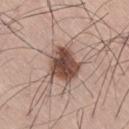  biopsy_status: not biopsied; imaged during a skin examination
  lesion_size:
    long_diameter_mm_approx: 4.0
  lighting: white-light
  patient:
    sex: male
    age_approx: 60
  site: leg
  image:
    source: total-body photography crop
    field_of_view_mm: 15
  automated_metrics:
    area_mm2_approx: 13.0
    shape_asymmetry: 0.25
    peripheral_color_asymmetry: 1.5